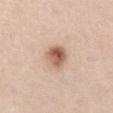Captured during whole-body skin photography for melanoma surveillance; the lesion was not biopsied. Located on the abdomen. This is a white-light tile. A male patient, aged around 65. Cropped from a whole-body photographic skin survey; the tile spans about 15 mm.